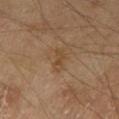{
  "biopsy_status": "not biopsied; imaged during a skin examination",
  "lighting": "cross-polarized",
  "lesion_size": {
    "long_diameter_mm_approx": 3.0
  },
  "patient": {
    "sex": "male",
    "age_approx": 70
  },
  "site": "left thigh",
  "image": {
    "source": "total-body photography crop",
    "field_of_view_mm": 15
  }
}